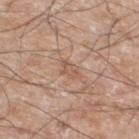Background:
The tile uses white-light illumination. From the left lower leg. The patient is a male roughly 60 years of age. A 15 mm close-up tile from a total-body photography series done for melanoma screening.